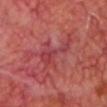biopsy_status: not biopsied; imaged during a skin examination
lesion_size:
  long_diameter_mm_approx: 6.0
patient:
  sex: male
  age_approx: 65
automated_metrics:
  area_mm2_approx: 9.0
  shape_asymmetry: 0.7
  vs_skin_darker_L: 6.0
  border_irregularity_0_10: 10.0
  color_variation_0_10: 3.0
  lesion_detection_confidence_0_100: 70
lighting: cross-polarized
site: head or neck
image:
  source: total-body photography crop
  field_of_view_mm: 15